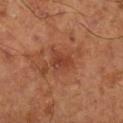From the right lower leg. A roughly 15 mm field-of-view crop from a total-body skin photograph. A male subject, aged approximately 65.